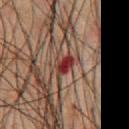lighting — cross-polarized illumination
size — ≈2.5 mm
acquisition — 15 mm crop, total-body photography
subject — male, approximately 65 years of age
body site — the chest